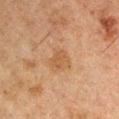Clinical impression:
Part of a total-body skin-imaging series; this lesion was reviewed on a skin check and was not flagged for biopsy.
Image and clinical context:
Imaged with cross-polarized lighting. A lesion tile, about 15 mm wide, cut from a 3D total-body photograph. The lesion is on the left upper arm. Longest diameter approximately 2.5 mm. A male subject, aged 48–52. Automated tile analysis of the lesion measured a lesion area of about 4 mm², an eccentricity of roughly 0.65, and two-axis asymmetry of about 0.25.